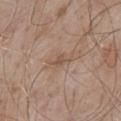Imaged during a routine full-body skin examination; the lesion was not biopsied and no histopathology is available. Imaged with white-light lighting. Approximately 3 mm at its widest. The patient is a male in their mid-50s. On the mid back. Cropped from a whole-body photographic skin survey; the tile spans about 15 mm.